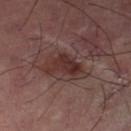No biopsy was performed on this lesion — it was imaged during a full skin examination and was not determined to be concerning.
This image is a 15 mm lesion crop taken from a total-body photograph.
Located on the left thigh.
This is a cross-polarized tile.
An algorithmic analysis of the crop reported an area of roughly 7 mm² and an eccentricity of roughly 0.75. It also reported a mean CIELAB color near L≈31 a*≈21 b*≈20, roughly 9 lightness units darker than nearby skin, and a normalized lesion–skin contrast near 9.
The recorded lesion diameter is about 4 mm.